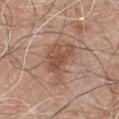<record>
<biopsy_status>not biopsied; imaged during a skin examination</biopsy_status>
<site>chest</site>
<image>
  <source>total-body photography crop</source>
  <field_of_view_mm>15</field_of_view_mm>
</image>
<automated_metrics>
  <eccentricity>0.75</eccentricity>
  <shape_asymmetry>0.4</shape_asymmetry>
  <vs_skin_darker_L>9.0</vs_skin_darker_L>
  <vs_skin_contrast_norm>6.5</vs_skin_contrast_norm>
  <nevus_likeness_0_100>10</nevus_likeness_0_100>
</automated_metrics>
<patient>
  <sex>male</sex>
  <age_approx>80</age_approx>
</patient>
</record>